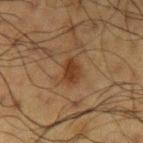workup: catalogued during a skin exam; not biopsied | tile lighting: cross-polarized illumination | body site: the left upper arm | acquisition: total-body-photography crop, ~15 mm field of view | diameter: about 3 mm | patient: male, about 60 years old | TBP lesion metrics: a footprint of about 5 mm²; a mean CIELAB color near L≈29 a*≈16 b*≈27; a border-irregularity rating of about 2.5/10 and internal color variation of about 2 on a 0–10 scale; a classifier nevus-likeness of about 85/100 and a detector confidence of about 100 out of 100 that the crop contains a lesion.